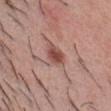No biopsy was performed on this lesion — it was imaged during a full skin examination and was not determined to be concerning. Located on the front of the torso. A male patient aged 33–37. This image is a 15 mm lesion crop taken from a total-body photograph.